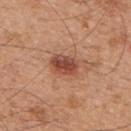Captured during whole-body skin photography for melanoma surveillance; the lesion was not biopsied. A roughly 15 mm field-of-view crop from a total-body skin photograph. The lesion is on the back. Captured under white-light illumination. The lesion-visualizer software estimated border irregularity of about 2.5 on a 0–10 scale, a within-lesion color-variation index near 5/10, and peripheral color asymmetry of about 1.5. The analysis additionally found an automated nevus-likeness rating near 90 out of 100 and lesion-presence confidence of about 100/100. A male patient, in their mid- to late 40s. Approximately 4.5 mm at its widest.